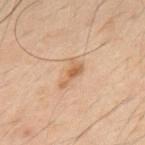The lesion was photographed on a routine skin check and not biopsied; there is no pathology result.
Approximately 3.5 mm at its widest.
Cropped from a total-body skin-imaging series; the visible field is about 15 mm.
Automated tile analysis of the lesion measured a normalized border contrast of about 7. It also reported an automated nevus-likeness rating near 25 out of 100.
A male patient aged 48–52.
The lesion is located on the upper back.